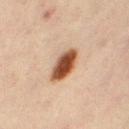On the right thigh. The subject is a female aged 43–47. The lesion-visualizer software estimated an average lesion color of about L≈44 a*≈21 b*≈31 (CIELAB), about 18 CIELAB-L* units darker than the surrounding skin, and a normalized lesion–skin contrast near 13.5. A 15 mm close-up extracted from a 3D total-body photography capture.